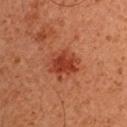Imaged during a routine full-body skin examination; the lesion was not biopsied and no histopathology is available.
A male patient aged approximately 50.
Cropped from a total-body skin-imaging series; the visible field is about 15 mm.
On the chest.